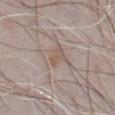Clinical impression: Recorded during total-body skin imaging; not selected for excision or biopsy. Context: The lesion is on the abdomen. A male subject aged 68–72. Measured at roughly 2.5 mm in maximum diameter. The lesion-visualizer software estimated a footprint of about 3 mm², an outline eccentricity of about 0.8 (0 = round, 1 = elongated), and a shape-asymmetry score of about 0.3 (0 = symmetric). And it measured an average lesion color of about L≈55 a*≈15 b*≈24 (CIELAB) and about 6 CIELAB-L* units darker than the surrounding skin. The analysis additionally found a peripheral color-asymmetry measure near 0. It also reported a nevus-likeness score of about 30/100 and a lesion-detection confidence of about 80/100. A 15 mm close-up tile from a total-body photography series done for melanoma screening. The tile uses white-light illumination.